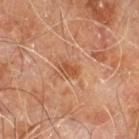The lesion was photographed on a routine skin check and not biopsied; there is no pathology result. Imaged with cross-polarized lighting. An algorithmic analysis of the crop reported a lesion–skin lightness drop of about 8 and a normalized lesion–skin contrast near 6.5. It also reported a color-variation rating of about 3/10 and radial color variation of about 1. This image is a 15 mm lesion crop taken from a total-body photograph. Located on the right leg. The patient is a male aged 58 to 62. Measured at roughly 2.5 mm in maximum diameter.